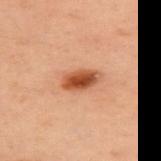| key | value |
|---|---|
| biopsy status | total-body-photography surveillance lesion; no biopsy |
| lighting | cross-polarized |
| size | ~4 mm (longest diameter) |
| image | ~15 mm crop, total-body skin-cancer survey |
| image-analysis metrics | a lesion area of about 6.5 mm² and a shape-asymmetry score of about 0.2 (0 = symmetric); an average lesion color of about L≈43 a*≈24 b*≈33 (CIELAB), a lesion–skin lightness drop of about 14, and a normalized lesion–skin contrast near 10.5; an automated nevus-likeness rating near 100 out of 100 and lesion-presence confidence of about 100/100 |
| patient | female, aged 53 to 57 |
| body site | the upper back |